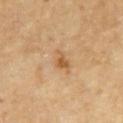Case summary:
• image: ~15 mm crop, total-body skin-cancer survey
• lighting: cross-polarized illumination
• body site: the back
• subject: male, in their mid- to late 80s or older
• automated metrics: a lesion color around L≈55 a*≈20 b*≈39 in CIELAB, about 9 CIELAB-L* units darker than the surrounding skin, and a normalized border contrast of about 7; lesion-presence confidence of about 100/100